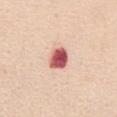Q: Was a biopsy performed?
A: no biopsy performed (imaged during a skin exam)
Q: What is the imaging modality?
A: ~15 mm tile from a whole-body skin photo
Q: Automated lesion metrics?
A: a border-irregularity rating of about 2/10 and peripheral color asymmetry of about 1.5
Q: Who is the patient?
A: female, in their mid-50s
Q: Where on the body is the lesion?
A: the chest
Q: Illumination type?
A: white-light illumination